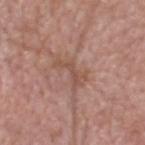| field | value |
|---|---|
| follow-up | total-body-photography surveillance lesion; no biopsy |
| TBP lesion metrics | a lesion area of about 5.5 mm², an outline eccentricity of about 0.85 (0 = round, 1 = elongated), and a shape-asymmetry score of about 0.6 (0 = symmetric); internal color variation of about 1.5 on a 0–10 scale; a detector confidence of about 80 out of 100 that the crop contains a lesion |
| image source | ~15 mm tile from a whole-body skin photo |
| size | ~4 mm (longest diameter) |
| subject | male, aged around 70 |
| site | the head or neck |
| illumination | white-light illumination |